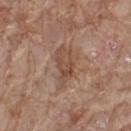Findings:
– notes · imaged on a skin check; not biopsied
– illumination · white-light
– anatomic site · the left thigh
– image source · total-body-photography crop, ~15 mm field of view
– size · ≈5 mm
– subject · male, aged 78 to 82